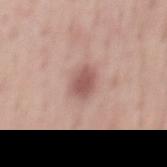Impression: The lesion was photographed on a routine skin check and not biopsied; there is no pathology result. Context: The lesion is on the mid back. This is a white-light tile. A region of skin cropped from a whole-body photographic capture, roughly 15 mm wide. A male subject about 55 years old. Measured at roughly 3.5 mm in maximum diameter. Automated image analysis of the tile measured a footprint of about 6 mm². The software also gave an automated nevus-likeness rating near 85 out of 100 and a lesion-detection confidence of about 100/100.